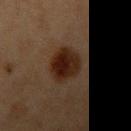Q: What lighting was used for the tile?
A: cross-polarized illumination
Q: What did automated image analysis measure?
A: a footprint of about 12 mm²; a lesion color around L≈19 a*≈16 b*≈22 in CIELAB and a normalized border contrast of about 12.5; an automated nevus-likeness rating near 100 out of 100
Q: How was this image acquired?
A: ~15 mm crop, total-body skin-cancer survey
Q: What are the patient's age and sex?
A: female, roughly 40 years of age
Q: Lesion location?
A: the left upper arm